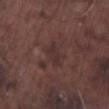{"automated_metrics": {"area_mm2_approx": 3.5, "eccentricity": 0.9, "shape_asymmetry": 0.35, "nevus_likeness_0_100": 0, "lesion_detection_confidence_0_100": 95}, "image": {"source": "total-body photography crop", "field_of_view_mm": 15}, "lighting": "white-light", "site": "right lower leg", "patient": {"sex": "male", "age_approx": 75}}